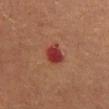Notes:
* workup — catalogued during a skin exam; not biopsied
* subject — female, aged 68–72
* TBP lesion metrics — border irregularity of about 2.5 on a 0–10 scale, a within-lesion color-variation index near 3/10, and radial color variation of about 1; a classifier nevus-likeness of about 0/100 and a lesion-detection confidence of about 100/100
* diameter — ~3 mm (longest diameter)
* imaging modality — ~15 mm crop, total-body skin-cancer survey
* illumination — cross-polarized illumination
* anatomic site — the chest